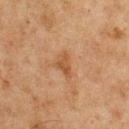From the chest.
A 15 mm close-up tile from a total-body photography series done for melanoma screening.
The patient is a male about 75 years old.